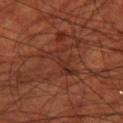The lesion was photographed on a routine skin check and not biopsied; there is no pathology result.
Automated image analysis of the tile measured a lesion area of about 8 mm², a shape eccentricity near 0.85, and a shape-asymmetry score of about 0.65 (0 = symmetric). The analysis additionally found roughly 5 lightness units darker than nearby skin. The analysis additionally found a classifier nevus-likeness of about 0/100 and a lesion-detection confidence of about 60/100.
Imaged with cross-polarized lighting.
A male patient, approximately 70 years of age.
The lesion is on the right thigh.
This image is a 15 mm lesion crop taken from a total-body photograph.